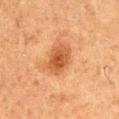Assessment:
No biopsy was performed on this lesion — it was imaged during a full skin examination and was not determined to be concerning.
Acquisition and patient details:
The lesion is on the right thigh. A region of skin cropped from a whole-body photographic capture, roughly 15 mm wide. A male subject, roughly 75 years of age. The recorded lesion diameter is about 3.5 mm.